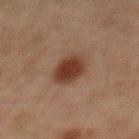{"lesion_size": {"long_diameter_mm_approx": 3.5}, "site": "front of the torso", "patient": {"sex": "male", "age_approx": 75}, "image": {"source": "total-body photography crop", "field_of_view_mm": 15}, "lighting": "cross-polarized"}